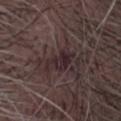Imaged during a routine full-body skin examination; the lesion was not biopsied and no histopathology is available.